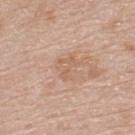{
  "biopsy_status": "not biopsied; imaged during a skin examination",
  "patient": {
    "sex": "male",
    "age_approx": 80
  },
  "image": {
    "source": "total-body photography crop",
    "field_of_view_mm": 15
  },
  "site": "upper back"
}